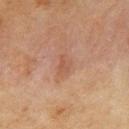This lesion was catalogued during total-body skin photography and was not selected for biopsy.
Located on the arm.
The subject is a female in their mid-50s.
A lesion tile, about 15 mm wide, cut from a 3D total-body photograph.
Approximately 2.5 mm at its widest.